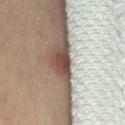anatomic site: the left thigh
TBP lesion metrics: an area of roughly 4.5 mm², a shape eccentricity near 0.9, and two-axis asymmetry of about 0.4; a lesion color around L≈45 a*≈20 b*≈23 in CIELAB, about 21 CIELAB-L* units darker than the surrounding skin, and a lesion-to-skin contrast of about 14.5 (normalized; higher = more distinct); lesion-presence confidence of about 100/100
acquisition: ~15 mm tile from a whole-body skin photo
tile lighting: cross-polarized